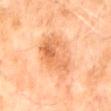Impression:
This lesion was catalogued during total-body skin photography and was not selected for biopsy.
Acquisition and patient details:
A roughly 15 mm field-of-view crop from a total-body skin photograph. A male subject, aged 58–62. Measured at roughly 7 mm in maximum diameter. On the abdomen. Captured under cross-polarized illumination.